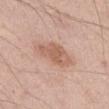<record>
  <lighting>white-light</lighting>
  <patient>
    <sex>male</sex>
    <age_approx>55</age_approx>
  </patient>
  <image>
    <source>total-body photography crop</source>
    <field_of_view_mm>15</field_of_view_mm>
  </image>
  <lesion_size>
    <long_diameter_mm_approx>5.0</long_diameter_mm_approx>
  </lesion_size>
  <site>abdomen</site>
</record>